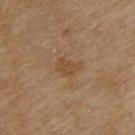  biopsy_status: not biopsied; imaged during a skin examination
  patient:
    sex: female
    age_approx: 65
  image:
    source: total-body photography crop
    field_of_view_mm: 15
  lighting: cross-polarized
  site: upper back